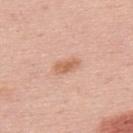Assessment: The lesion was tiled from a total-body skin photograph and was not biopsied. Image and clinical context: Automated image analysis of the tile measured a border-irregularity rating of about 1.5/10 and a peripheral color-asymmetry measure near 0.5. The analysis additionally found an automated nevus-likeness rating near 15 out of 100. The lesion is on the upper back. About 3 mm across. A 15 mm crop from a total-body photograph taken for skin-cancer surveillance. A male subject, aged 63 to 67.